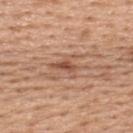Case summary:
* notes — total-body-photography surveillance lesion; no biopsy